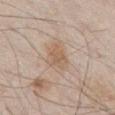biopsy status: catalogued during a skin exam; not biopsied
image source: total-body-photography crop, ~15 mm field of view
anatomic site: the chest
patient: male, in their 80s